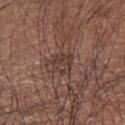Impression: Part of a total-body skin-imaging series; this lesion was reviewed on a skin check and was not flagged for biopsy. Context: A male patient, roughly 65 years of age. The total-body-photography lesion software estimated a border-irregularity rating of about 3/10 and peripheral color asymmetry of about 1. On the arm. This is a white-light tile. A region of skin cropped from a whole-body photographic capture, roughly 15 mm wide. Longest diameter approximately 3 mm.